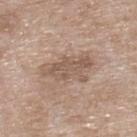follow-up — no biopsy performed (imaged during a skin exam)
diameter — ~5.5 mm (longest diameter)
patient — female, aged 73 to 77
body site — the upper back
lighting — white-light illumination
image source — ~15 mm tile from a whole-body skin photo